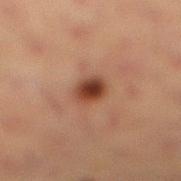Q: Is there a histopathology result?
A: catalogued during a skin exam; not biopsied
Q: Lesion location?
A: the right lower leg
Q: What did automated image analysis measure?
A: an area of roughly 6 mm², a shape eccentricity near 0.65, and a symmetry-axis asymmetry near 0.2; a lesion–skin lightness drop of about 12 and a normalized border contrast of about 10.5
Q: Who is the patient?
A: female, approximately 40 years of age
Q: How was the tile lit?
A: cross-polarized
Q: How was this image acquired?
A: ~15 mm crop, total-body skin-cancer survey
Q: Lesion size?
A: ~3 mm (longest diameter)